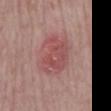– size · ≈5.5 mm
– patient · male, in their 60s
– lighting · white-light illumination
– imaging modality · ~15 mm tile from a whole-body skin photo
– site · the mid back
– histopathology · a nodular basal cell carcinoma (malignant)